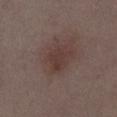Notes:
– biopsy status · total-body-photography surveillance lesion; no biopsy
– patient · female, approximately 35 years of age
– acquisition · ~15 mm tile from a whole-body skin photo
– illumination · white-light illumination
– site · the right thigh
– diameter · about 3.5 mm